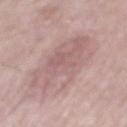{
  "biopsy_status": "not biopsied; imaged during a skin examination",
  "image": {
    "source": "total-body photography crop",
    "field_of_view_mm": 15
  },
  "patient": {
    "sex": "male",
    "age_approx": 65
  },
  "site": "mid back"
}